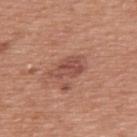No biopsy was performed on this lesion — it was imaged during a full skin examination and was not determined to be concerning.
A 15 mm close-up extracted from a 3D total-body photography capture.
Located on the upper back.
This is a white-light tile.
Longest diameter approximately 4 mm.
A male patient, aged approximately 75.
An algorithmic analysis of the crop reported a footprint of about 7 mm² and a shape-asymmetry score of about 0.35 (0 = symmetric). It also reported an average lesion color of about L≈50 a*≈25 b*≈27 (CIELAB), roughly 9 lightness units darker than nearby skin, and a normalized lesion–skin contrast near 7. And it measured an automated nevus-likeness rating near 45 out of 100 and a detector confidence of about 100 out of 100 that the crop contains a lesion.